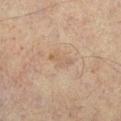This lesion was catalogued during total-body skin photography and was not selected for biopsy. This image is a 15 mm lesion crop taken from a total-body photograph. A male patient, about 75 years old. On the right lower leg.